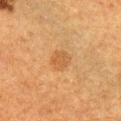follow-up: no biopsy performed (imaged during a skin exam) | TBP lesion metrics: an outline eccentricity of about 0.5 (0 = round, 1 = elongated); a mean CIELAB color near L≈46 a*≈19 b*≈35, roughly 6 lightness units darker than nearby skin, and a normalized border contrast of about 5.5; an automated nevus-likeness rating near 55 out of 100 and a detector confidence of about 100 out of 100 that the crop contains a lesion | acquisition: total-body-photography crop, ~15 mm field of view | body site: the right upper arm | patient: male, about 50 years old | diameter: ≈3 mm | tile lighting: cross-polarized illumination.